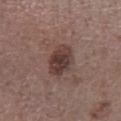<record>
  <biopsy_status>not biopsied; imaged during a skin examination</biopsy_status>
  <lesion_size>
    <long_diameter_mm_approx>4.5</long_diameter_mm_approx>
  </lesion_size>
  <automated_metrics>
    <area_mm2_approx>10.0</area_mm2_approx>
    <eccentricity>0.75</eccentricity>
    <shape_asymmetry>0.15</shape_asymmetry>
    <cielab_L>38</cielab_L>
    <cielab_a>17</cielab_a>
    <cielab_b>20</cielab_b>
    <vs_skin_contrast_norm>9.5</vs_skin_contrast_norm>
  </automated_metrics>
  <image>
    <source>total-body photography crop</source>
    <field_of_view_mm>15</field_of_view_mm>
  </image>
  <site>left lower leg</site>
  <lighting>white-light</lighting>
  <patient>
    <sex>male</sex>
    <age_approx>45</age_approx>
  </patient>
</record>